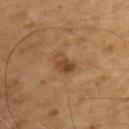follow-up = imaged on a skin check; not biopsied | tile lighting = cross-polarized illumination | patient = male, about 55 years old | TBP lesion metrics = a lesion-detection confidence of about 100/100 | diameter = about 3 mm | site = the upper back | acquisition = ~15 mm crop, total-body skin-cancer survey.